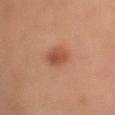Part of a total-body skin-imaging series; this lesion was reviewed on a skin check and was not flagged for biopsy. A 15 mm crop from a total-body photograph taken for skin-cancer surveillance. Automated tile analysis of the lesion measured an average lesion color of about L≈41 a*≈22 b*≈27 (CIELAB) and a lesion–skin lightness drop of about 9. It also reported a detector confidence of about 100 out of 100 that the crop contains a lesion. The tile uses cross-polarized illumination. A female patient, in their mid-50s. Located on the head or neck. The lesion's longest dimension is about 2.5 mm.